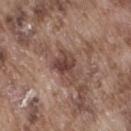| feature | finding |
|---|---|
| biopsy status | catalogued during a skin exam; not biopsied |
| subject | male, about 75 years old |
| TBP lesion metrics | an area of roughly 8 mm² and an eccentricity of roughly 0.75; a mean CIELAB color near L≈43 a*≈19 b*≈23 and a normalized border contrast of about 8.5; border irregularity of about 4 on a 0–10 scale and a color-variation rating of about 4.5/10 |
| lesion size | ≈4 mm |
| imaging modality | total-body-photography crop, ~15 mm field of view |
| body site | the right thigh |
| tile lighting | white-light |